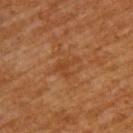Q: Was a biopsy performed?
A: total-body-photography surveillance lesion; no biopsy
Q: Lesion size?
A: ~3 mm (longest diameter)
Q: What did automated image analysis measure?
A: roughly 6 lightness units darker than nearby skin; internal color variation of about 2 on a 0–10 scale
Q: What is the anatomic site?
A: the upper back
Q: Who is the patient?
A: male, roughly 60 years of age
Q: How was this image acquired?
A: ~15 mm tile from a whole-body skin photo
Q: Illumination type?
A: cross-polarized